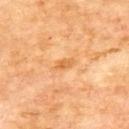biopsy_status: not biopsied; imaged during a skin examination
lesion_size:
  long_diameter_mm_approx: 2.5
lighting: cross-polarized
image:
  source: total-body photography crop
  field_of_view_mm: 15
patient:
  sex: male
  age_approx: 70
site: upper back
automated_metrics:
  border_irregularity_0_10: 3.0
  color_variation_0_10: 3.0
  peripheral_color_asymmetry: 1.0
  nevus_likeness_0_100: 0
  lesion_detection_confidence_0_100: 100